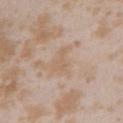Clinical impression:
Recorded during total-body skin imaging; not selected for excision or biopsy.
Context:
Located on the left upper arm. A female subject, in their mid- to late 20s. A region of skin cropped from a whole-body photographic capture, roughly 15 mm wide. Imaged with white-light lighting. The total-body-photography lesion software estimated a lesion area of about 4 mm², a shape eccentricity near 0.85, and two-axis asymmetry of about 0.55.